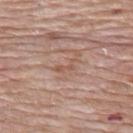<case>
  <biopsy_status>not biopsied; imaged during a skin examination</biopsy_status>
  <lighting>white-light</lighting>
  <site>upper back</site>
  <patient>
    <sex>female</sex>
    <age_approx>65</age_approx>
  </patient>
  <lesion_size>
    <long_diameter_mm_approx>3.0</long_diameter_mm_approx>
  </lesion_size>
  <image>
    <source>total-body photography crop</source>
    <field_of_view_mm>15</field_of_view_mm>
  </image>
</case>